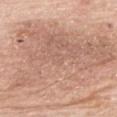follow-up: total-body-photography surveillance lesion; no biopsy | acquisition: 15 mm crop, total-body photography | patient: male, aged 78 to 82 | site: the mid back.